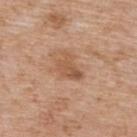| key | value |
|---|---|
| follow-up | catalogued during a skin exam; not biopsied |